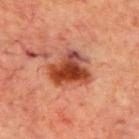workup = imaged on a skin check; not biopsied
TBP lesion metrics = a lesion area of about 16 mm², a shape eccentricity near 0.5, and two-axis asymmetry of about 0.3; an automated nevus-likeness rating near 95 out of 100 and a lesion-detection confidence of about 100/100
subject = male, roughly 70 years of age
imaging modality = 15 mm crop, total-body photography
site = the back
illumination = cross-polarized
lesion diameter = ~5.5 mm (longest diameter)